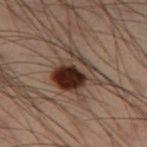lighting: cross-polarized illumination | automated lesion analysis: a footprint of about 14 mm² and an outline eccentricity of about 0.6 (0 = round, 1 = elongated); a classifier nevus-likeness of about 95/100 and a detector confidence of about 100 out of 100 that the crop contains a lesion | anatomic site: the left thigh | acquisition: ~15 mm tile from a whole-body skin photo | patient: male, in their mid-50s | lesion size: ≈4.5 mm.